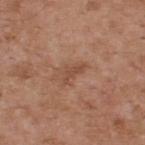The lesion was tiled from a total-body skin photograph and was not biopsied. The subject is a male aged around 55. Automated tile analysis of the lesion measured a mean CIELAB color near L≈48 a*≈22 b*≈31, roughly 8 lightness units darker than nearby skin, and a normalized border contrast of about 6. It also reported border irregularity of about 6.5 on a 0–10 scale, a within-lesion color-variation index near 0/10, and peripheral color asymmetry of about 0. The software also gave a nevus-likeness score of about 0/100 and a detector confidence of about 100 out of 100 that the crop contains a lesion. This is a white-light tile. Located on the upper back. Cropped from a whole-body photographic skin survey; the tile spans about 15 mm. The recorded lesion diameter is about 3 mm.